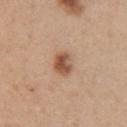workup: total-body-photography surveillance lesion; no biopsy
imaging modality: ~15 mm crop, total-body skin-cancer survey
image-analysis metrics: an average lesion color of about L≈54 a*≈20 b*≈32 (CIELAB) and about 13 CIELAB-L* units darker than the surrounding skin; an automated nevus-likeness rating near 95 out of 100 and a detector confidence of about 100 out of 100 that the crop contains a lesion
lesion diameter: ~3 mm (longest diameter)
subject: female, approximately 30 years of age
illumination: white-light
site: the chest